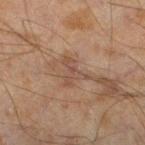biopsy status: no biopsy performed (imaged during a skin exam)
size: ~3.5 mm (longest diameter)
automated lesion analysis: a footprint of about 5 mm², a shape eccentricity near 0.65, and a shape-asymmetry score of about 0.65 (0 = symmetric)
tile lighting: cross-polarized illumination
patient: male, approximately 45 years of age
location: the left lower leg
image: ~15 mm tile from a whole-body skin photo